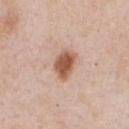The lesion was tiled from a total-body skin photograph and was not biopsied.
The lesion is located on the chest.
The subject is a male about 60 years old.
About 4 mm across.
A 15 mm close-up tile from a total-body photography series done for melanoma screening.